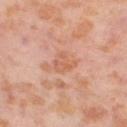Recorded during total-body skin imaging; not selected for excision or biopsy. A female subject, approximately 55 years of age. A lesion tile, about 15 mm wide, cut from a 3D total-body photograph. From the right thigh. Approximately 3 mm at its widest. The total-body-photography lesion software estimated a lesion area of about 5.5 mm², a shape eccentricity near 0.4, and a shape-asymmetry score of about 0.35 (0 = symmetric). The software also gave a lesion-to-skin contrast of about 5 (normalized; higher = more distinct). It also reported a border-irregularity rating of about 3.5/10, internal color variation of about 3.5 on a 0–10 scale, and radial color variation of about 1.5. And it measured a classifier nevus-likeness of about 0/100.